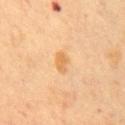Q: Was a biopsy performed?
A: catalogued during a skin exam; not biopsied
Q: What did automated image analysis measure?
A: a mean CIELAB color near L≈61 a*≈19 b*≈41 and roughly 8 lightness units darker than nearby skin
Q: Illumination type?
A: cross-polarized illumination
Q: What are the patient's age and sex?
A: female, aged 58–62
Q: Lesion size?
A: ~2.5 mm (longest diameter)
Q: Where on the body is the lesion?
A: the chest
Q: What is the imaging modality?
A: ~15 mm tile from a whole-body skin photo